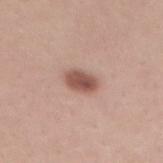  biopsy_status: not biopsied; imaged during a skin examination